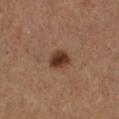Captured during whole-body skin photography for melanoma surveillance; the lesion was not biopsied.
The lesion is on the leg.
The patient is a male aged 63–67.
A lesion tile, about 15 mm wide, cut from a 3D total-body photograph.
Longest diameter approximately 2.5 mm.
This is a cross-polarized tile.